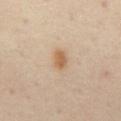Assessment: Recorded during total-body skin imaging; not selected for excision or biopsy. Clinical summary: About 2.5 mm across. The subject is a male about 55 years old. A region of skin cropped from a whole-body photographic capture, roughly 15 mm wide. The lesion is located on the abdomen. Captured under cross-polarized illumination.